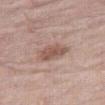| feature | finding |
|---|---|
| follow-up | imaged on a skin check; not biopsied |
| patient | male, aged 78 to 82 |
| image | total-body-photography crop, ~15 mm field of view |
| lighting | white-light illumination |
| location | the right thigh |
| diameter | ~4 mm (longest diameter) |